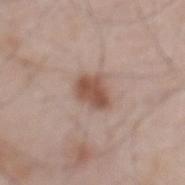Part of a total-body skin-imaging series; this lesion was reviewed on a skin check and was not flagged for biopsy.
The patient is a male aged approximately 65.
A lesion tile, about 15 mm wide, cut from a 3D total-body photograph.
Captured under white-light illumination.
Longest diameter approximately 3.5 mm.
The lesion is located on the mid back.